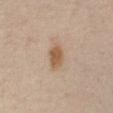| feature | finding |
|---|---|
| biopsy status | imaged on a skin check; not biopsied |
| patient | male, about 65 years old |
| body site | the abdomen |
| image source | ~15 mm tile from a whole-body skin photo |
| tile lighting | cross-polarized illumination |